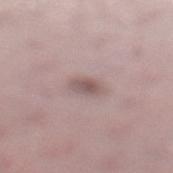workup = total-body-photography surveillance lesion; no biopsy
illumination = white-light
size = about 2.5 mm
patient = female, aged around 45
image-analysis metrics = a mean CIELAB color near L≈54 a*≈17 b*≈18
anatomic site = the left lower leg
image = 15 mm crop, total-body photography